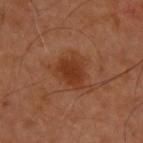Part of a total-body skin-imaging series; this lesion was reviewed on a skin check and was not flagged for biopsy. A roughly 15 mm field-of-view crop from a total-body skin photograph. Imaged with cross-polarized lighting. The recorded lesion diameter is about 4 mm. Located on the upper back. A male subject, in their mid-50s.